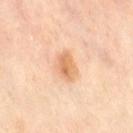No biopsy was performed on this lesion — it was imaged during a full skin examination and was not determined to be concerning. The subject is a female roughly 50 years of age. The lesion-visualizer software estimated a border-irregularity index near 2/10 and a within-lesion color-variation index near 4/10. A 15 mm crop from a total-body photograph taken for skin-cancer surveillance. The lesion is on the right thigh. Measured at roughly 3.5 mm in maximum diameter. Imaged with cross-polarized lighting.